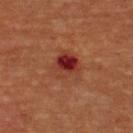notes: no biopsy performed (imaged during a skin exam) | body site: the chest | patient: male, in their mid- to late 60s | automated metrics: a lesion color around L≈33 a*≈27 b*≈28 in CIELAB, a lesion–skin lightness drop of about 9, and a lesion-to-skin contrast of about 9 (normalized; higher = more distinct); a border-irregularity index near 2.5/10, a color-variation rating of about 10/10, and peripheral color asymmetry of about 3.5 | lighting: cross-polarized | diameter: about 3.5 mm | image: ~15 mm crop, total-body skin-cancer survey.